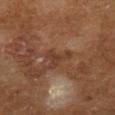The lesion is on the leg.
A male patient, aged approximately 65.
Cropped from a whole-body photographic skin survey; the tile spans about 15 mm.